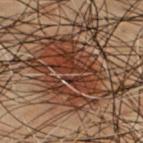• notes · catalogued during a skin exam; not biopsied
• image · ~15 mm crop, total-body skin-cancer survey
• subject · male, in their mid- to late 50s
• site · the chest
• lighting · cross-polarized
• TBP lesion metrics · a shape eccentricity near 0.8; a lesion color around L≈30 a*≈17 b*≈24 in CIELAB, about 10 CIELAB-L* units darker than the surrounding skin, and a lesion-to-skin contrast of about 9.5 (normalized; higher = more distinct)